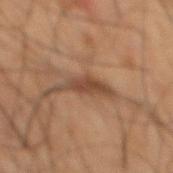Notes:
• workup: imaged on a skin check; not biopsied
• diameter: about 5 mm
• body site: the mid back
• acquisition: ~15 mm crop, total-body skin-cancer survey
• subject: male, in their 50s
• illumination: cross-polarized
• automated lesion analysis: a classifier nevus-likeness of about 50/100 and a detector confidence of about 55 out of 100 that the crop contains a lesion